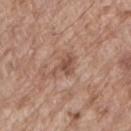Notes:
– notes: total-body-photography surveillance lesion; no biopsy
– anatomic site: the lower back
– lesion diameter: ~3.5 mm (longest diameter)
– image: ~15 mm crop, total-body skin-cancer survey
– subject: male, approximately 80 years of age
– illumination: white-light